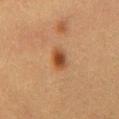The lesion was photographed on a routine skin check and not biopsied; there is no pathology result. Located on the chest. This image is a 15 mm lesion crop taken from a total-body photograph. A female subject, aged approximately 40. This is a cross-polarized tile.